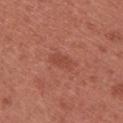Assessment: This lesion was catalogued during total-body skin photography and was not selected for biopsy. Background: From the upper back. The patient is a female aged 23–27. A close-up tile cropped from a whole-body skin photograph, about 15 mm across.